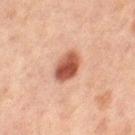Assessment:
The lesion was photographed on a routine skin check and not biopsied; there is no pathology result.
Context:
A roughly 15 mm field-of-view crop from a total-body skin photograph. From the left thigh. Automated tile analysis of the lesion measured a shape eccentricity near 0.7. The software also gave border irregularity of about 1.5 on a 0–10 scale and radial color variation of about 1.5. Longest diameter approximately 3.5 mm. Captured under cross-polarized illumination. The patient is a female aged 58 to 62.